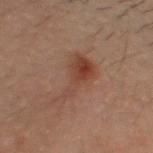follow-up — catalogued during a skin exam; not biopsied | subject — male, in their 30s | anatomic site — the head or neck | size — about 7 mm | image — ~15 mm tile from a whole-body skin photo.